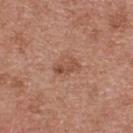notes: no biopsy performed (imaged during a skin exam) | illumination: white-light illumination | image: ~15 mm crop, total-body skin-cancer survey | lesion diameter: ~3.5 mm (longest diameter) | patient: female, aged 38–42 | site: the upper back.